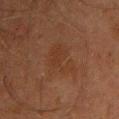<record>
<biopsy_status>not biopsied; imaged during a skin examination</biopsy_status>
<automated_metrics>
  <peripheral_color_asymmetry>0.5</peripheral_color_asymmetry>
  <nevus_likeness_0_100>0</nevus_likeness_0_100>
  <lesion_detection_confidence_0_100>100</lesion_detection_confidence_0_100>
</automated_metrics>
<lighting>cross-polarized</lighting>
<image>
  <source>total-body photography crop</source>
  <field_of_view_mm>15</field_of_view_mm>
</image>
<patient>
  <sex>male</sex>
  <age_approx>45</age_approx>
</patient>
<site>front of the torso</site>
</record>